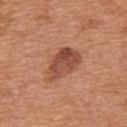Impression:
The lesion was tiled from a total-body skin photograph and was not biopsied.
Clinical summary:
A roughly 15 mm field-of-view crop from a total-body skin photograph. Captured under white-light illumination. On the mid back. The lesion's longest dimension is about 5 mm. A male patient, in their mid- to late 60s.